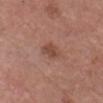Impression:
Part of a total-body skin-imaging series; this lesion was reviewed on a skin check and was not flagged for biopsy.
Background:
A 15 mm close-up tile from a total-body photography series done for melanoma screening. On the head or neck. A male patient aged approximately 80. Automated tile analysis of the lesion measured roughly 9 lightness units darker than nearby skin and a normalized border contrast of about 6.5. Captured under white-light illumination.